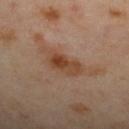Notes:
- follow-up · imaged on a skin check; not biopsied
- location · the upper back
- image · 15 mm crop, total-body photography
- subject · female, roughly 40 years of age